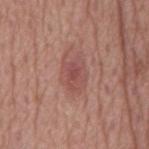The lesion was photographed on a routine skin check and not biopsied; there is no pathology result. The lesion-visualizer software estimated a border-irregularity index near 3/10 and a within-lesion color-variation index near 3/10. A roughly 15 mm field-of-view crop from a total-body skin photograph. On the mid back. The patient is a male about 75 years old.